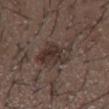{
  "biopsy_status": "not biopsied; imaged during a skin examination",
  "image": {
    "source": "total-body photography crop",
    "field_of_view_mm": 15
  },
  "lesion_size": {
    "long_diameter_mm_approx": 4.0
  },
  "site": "mid back",
  "patient": {
    "sex": "male",
    "age_approx": 50
  },
  "automated_metrics": {
    "area_mm2_approx": 11.0,
    "nevus_likeness_0_100": 5,
    "lesion_detection_confidence_0_100": 100
  }
}